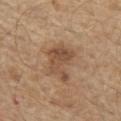Q: Is there a histopathology result?
A: imaged on a skin check; not biopsied
Q: What kind of image is this?
A: 15 mm crop, total-body photography
Q: Patient demographics?
A: male, aged 68 to 72
Q: Lesion location?
A: the front of the torso